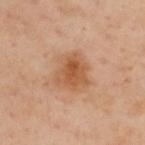From the back. A close-up tile cropped from a whole-body skin photograph, about 15 mm across. About 4 mm across. The subject is a male aged 53 to 57. The lesion-visualizer software estimated a lesion color around L≈55 a*≈23 b*≈36 in CIELAB, about 10 CIELAB-L* units darker than the surrounding skin, and a normalized border contrast of about 7.5. And it measured a nevus-likeness score of about 80/100. This is a cross-polarized tile.